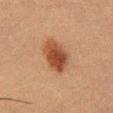Assessment:
The lesion was photographed on a routine skin check and not biopsied; there is no pathology result.
Clinical summary:
On the chest. A male subject, aged around 50. Imaged with cross-polarized lighting. A roughly 15 mm field-of-view crop from a total-body skin photograph.